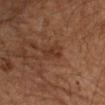workup=catalogued during a skin exam; not biopsied | lesion size=about 2.5 mm | tile lighting=cross-polarized | image=total-body-photography crop, ~15 mm field of view | patient=male, aged 48–52 | body site=the left upper arm.